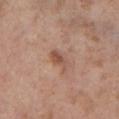Part of a total-body skin-imaging series; this lesion was reviewed on a skin check and was not flagged for biopsy.
The recorded lesion diameter is about 3 mm.
The patient is a male aged 53–57.
The tile uses white-light illumination.
A lesion tile, about 15 mm wide, cut from a 3D total-body photograph.
The lesion is located on the chest.